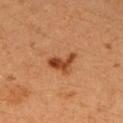| field | value |
|---|---|
| follow-up | imaged on a skin check; not biopsied |
| tile lighting | cross-polarized illumination |
| patient | female, roughly 40 years of age |
| diameter | ~5 mm (longest diameter) |
| image-analysis metrics | a border-irregularity index near 4.5/10, a within-lesion color-variation index near 8/10, and peripheral color asymmetry of about 3; a classifier nevus-likeness of about 75/100 and a detector confidence of about 100 out of 100 that the crop contains a lesion |
| location | the right upper arm |
| image source | 15 mm crop, total-body photography |